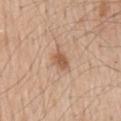Clinical impression:
Part of a total-body skin-imaging series; this lesion was reviewed on a skin check and was not flagged for biopsy.
Image and clinical context:
A 15 mm close-up tile from a total-body photography series done for melanoma screening. The recorded lesion diameter is about 2.5 mm. Captured under white-light illumination. A male patient, about 60 years old. From the mid back.